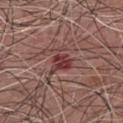Clinical summary: Cropped from a total-body skin-imaging series; the visible field is about 15 mm. This is a white-light tile. Approximately 3.5 mm at its widest. From the chest. A male subject aged 73 to 77.